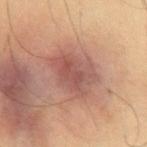Assessment:
Recorded during total-body skin imaging; not selected for excision or biopsy.
Acquisition and patient details:
This is a cross-polarized tile. The lesion-visualizer software estimated a classifier nevus-likeness of about 40/100 and lesion-presence confidence of about 100/100. The subject is a male in their mid- to late 50s. About 6 mm across. A lesion tile, about 15 mm wide, cut from a 3D total-body photograph. On the abdomen.